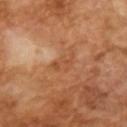The lesion was tiled from a total-body skin photograph and was not biopsied. A region of skin cropped from a whole-body photographic capture, roughly 15 mm wide. The tile uses cross-polarized illumination. A male subject roughly 65 years of age. Automated tile analysis of the lesion measured a border-irregularity index near 6.5/10, a color-variation rating of about 2/10, and a peripheral color-asymmetry measure near 0.5. Longest diameter approximately 4 mm.